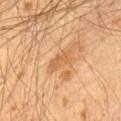Q: Is there a histopathology result?
A: total-body-photography surveillance lesion; no biopsy
Q: What is the anatomic site?
A: the front of the torso
Q: How was this image acquired?
A: total-body-photography crop, ~15 mm field of view
Q: Patient demographics?
A: male, approximately 55 years of age